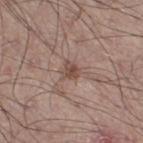workup: catalogued during a skin exam; not biopsied | automated lesion analysis: an area of roughly 4.5 mm², an eccentricity of roughly 0.65, and a shape-asymmetry score of about 0.45 (0 = symmetric); a lesion color around L≈49 a*≈17 b*≈23 in CIELAB, roughly 9 lightness units darker than nearby skin, and a normalized lesion–skin contrast near 6.5 | image: ~15 mm tile from a whole-body skin photo | lighting: white-light illumination | location: the right thigh | subject: male, about 50 years old.